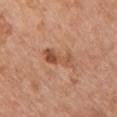Q: Was this lesion biopsied?
A: total-body-photography surveillance lesion; no biopsy
Q: How was the tile lit?
A: white-light illumination
Q: Lesion location?
A: the chest
Q: Who is the patient?
A: male, about 60 years old
Q: What did automated image analysis measure?
A: a nevus-likeness score of about 40/100
Q: What is the imaging modality?
A: ~15 mm crop, total-body skin-cancer survey
Q: How large is the lesion?
A: ~4 mm (longest diameter)